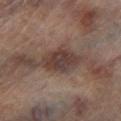<record>
<biopsy_status>not biopsied; imaged during a skin examination</biopsy_status>
<automated_metrics>
  <nevus_likeness_0_100>20</nevus_likeness_0_100>
</automated_metrics>
<patient>
  <sex>male</sex>
  <age_approx>65</age_approx>
</patient>
<site>left lower leg</site>
<lighting>cross-polarized</lighting>
<lesion_size>
  <long_diameter_mm_approx>4.5</long_diameter_mm_approx>
</lesion_size>
<image>
  <source>total-body photography crop</source>
  <field_of_view_mm>15</field_of_view_mm>
</image>
</record>